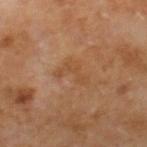follow-up: catalogued during a skin exam; not biopsied
imaging modality: ~15 mm crop, total-body skin-cancer survey
body site: the leg
tile lighting: cross-polarized illumination
patient: male, aged around 60
lesion size: ~4 mm (longest diameter)